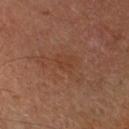Q: Was a biopsy performed?
A: catalogued during a skin exam; not biopsied
Q: How was this image acquired?
A: ~15 mm tile from a whole-body skin photo
Q: Where on the body is the lesion?
A: the right forearm
Q: What lighting was used for the tile?
A: cross-polarized
Q: How large is the lesion?
A: about 6 mm
Q: What are the patient's age and sex?
A: male, in their mid- to late 60s
Q: What did automated image analysis measure?
A: an eccentricity of roughly 0.85 and two-axis asymmetry of about 0.65; a lesion-detection confidence of about 100/100